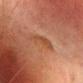The lesion was tiled from a total-body skin photograph and was not biopsied. The lesion is on the head or neck. Longest diameter approximately 3.5 mm. The patient is a female roughly 60 years of age. A 15 mm crop from a total-body photograph taken for skin-cancer surveillance.